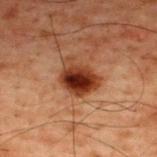| key | value |
|---|---|
| biopsy status | total-body-photography surveillance lesion; no biopsy |
| automated lesion analysis | an average lesion color of about L≈27 a*≈22 b*≈27 (CIELAB), about 14 CIELAB-L* units darker than the surrounding skin, and a lesion-to-skin contrast of about 13.5 (normalized; higher = more distinct) |
| subject | male, aged 58–62 |
| acquisition | total-body-photography crop, ~15 mm field of view |
| site | the upper back |
| lighting | cross-polarized |